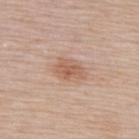The lesion was photographed on a routine skin check and not biopsied; there is no pathology result.
The total-body-photography lesion software estimated about 9 CIELAB-L* units darker than the surrounding skin and a lesion-to-skin contrast of about 6.5 (normalized; higher = more distinct).
The subject is a female roughly 65 years of age.
About 4 mm across.
A close-up tile cropped from a whole-body skin photograph, about 15 mm across.
From the upper back.
Captured under white-light illumination.